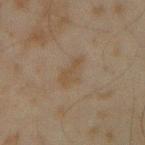  biopsy_status: not biopsied; imaged during a skin examination
  site: right forearm
  image:
    source: total-body photography crop
    field_of_view_mm: 15
  patient:
    sex: male
    age_approx: 45